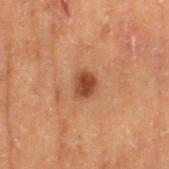| feature | finding |
|---|---|
| notes | imaged on a skin check; not biopsied |
| lighting | cross-polarized illumination |
| TBP lesion metrics | a classifier nevus-likeness of about 95/100 and a lesion-detection confidence of about 100/100 |
| imaging modality | total-body-photography crop, ~15 mm field of view |
| subject | male, in their 70s |
| lesion diameter | about 3 mm |
| body site | the leg |